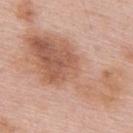Assessment: Imaged during a routine full-body skin examination; the lesion was not biopsied and no histopathology is available. Clinical summary: A roughly 15 mm field-of-view crop from a total-body skin photograph. From the upper back. The tile uses white-light illumination. A male subject, roughly 55 years of age.